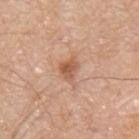The lesion was tiled from a total-body skin photograph and was not biopsied. This is a white-light tile. Located on the upper back. The recorded lesion diameter is about 3 mm. A male patient, roughly 80 years of age. A roughly 15 mm field-of-view crop from a total-body skin photograph.